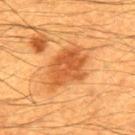| field | value |
|---|---|
| biopsy status | no biopsy performed (imaged during a skin exam) |
| subject | male, about 60 years old |
| site | the mid back |
| image | 15 mm crop, total-body photography |